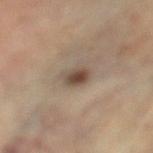The lesion was tiled from a total-body skin photograph and was not biopsied.
The lesion's longest dimension is about 3 mm.
A female patient, aged 48 to 52.
Captured under cross-polarized illumination.
Automated tile analysis of the lesion measured an area of roughly 4.5 mm² and two-axis asymmetry of about 0.25. And it measured a border-irregularity rating of about 2.5/10, a color-variation rating of about 5/10, and radial color variation of about 1.5. The software also gave lesion-presence confidence of about 100/100.
A 15 mm close-up tile from a total-body photography series done for melanoma screening.
On the left thigh.